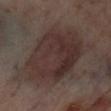The lesion was tiled from a total-body skin photograph and was not biopsied. On the left lower leg. The subject is a female aged approximately 55. A roughly 15 mm field-of-view crop from a total-body skin photograph. Imaged with cross-polarized lighting. Longest diameter approximately 12 mm.